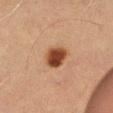notes = total-body-photography surveillance lesion; no biopsy | image source = ~15 mm tile from a whole-body skin photo | location = the left thigh | automated metrics = a footprint of about 7.5 mm² and two-axis asymmetry of about 0.15 | subject = male, aged around 70 | size = ≈3.5 mm | tile lighting = cross-polarized illumination.